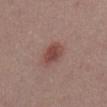notes: catalogued during a skin exam; not biopsied | anatomic site: the abdomen | subject: male, about 55 years old | lesion diameter: about 3.5 mm | imaging modality: 15 mm crop, total-body photography.